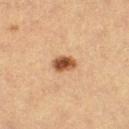  biopsy_status: not biopsied; imaged during a skin examination
  image:
    source: total-body photography crop
    field_of_view_mm: 15
  site: left thigh
  patient:
    sex: female
    age_approx: 30